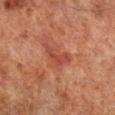Impression: No biopsy was performed on this lesion — it was imaged during a full skin examination and was not determined to be concerning. Acquisition and patient details: The lesion's longest dimension is about 3.5 mm. The lesion is located on the right lower leg. A male subject approximately 70 years of age. This is a cross-polarized tile. A 15 mm close-up tile from a total-body photography series done for melanoma screening.